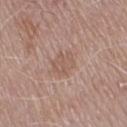Case summary:
* biopsy status — total-body-photography surveillance lesion; no biopsy
* anatomic site — the chest
* patient — male, in their mid-70s
* image-analysis metrics — a footprint of about 5.5 mm² and two-axis asymmetry of about 0.2; roughly 6 lightness units darker than nearby skin and a normalized lesion–skin contrast near 4.5; a lesion-detection confidence of about 100/100
* image source — total-body-photography crop, ~15 mm field of view
* lighting — white-light